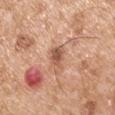Part of a total-body skin-imaging series; this lesion was reviewed on a skin check and was not flagged for biopsy.
This is a white-light tile.
A roughly 15 mm field-of-view crop from a total-body skin photograph.
A male subject roughly 55 years of age.
The recorded lesion diameter is about 3 mm.
The lesion is on the left upper arm.